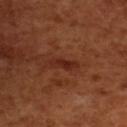Part of a total-body skin-imaging series; this lesion was reviewed on a skin check and was not flagged for biopsy.
This is a cross-polarized tile.
Approximately 3.5 mm at its widest.
A female subject, aged approximately 55.
The lesion is located on the upper back.
A roughly 15 mm field-of-view crop from a total-body skin photograph.